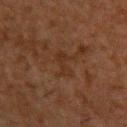biopsy status = total-body-photography surveillance lesion; no biopsy | body site = the upper back | acquisition = 15 mm crop, total-body photography | patient = male, aged 48 to 52.